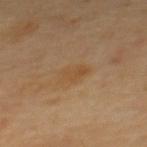This lesion was catalogued during total-body skin photography and was not selected for biopsy. Located on the mid back. About 3.5 mm across. The patient is a male in their 60s. A 15 mm close-up tile from a total-body photography series done for melanoma screening.